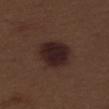{"biopsy_status": "not biopsied; imaged during a skin examination", "site": "right thigh", "patient": {"sex": "male", "age_approx": 70}, "automated_metrics": {"nevus_likeness_0_100": 90}, "image": {"source": "total-body photography crop", "field_of_view_mm": 15}, "lesion_size": {"long_diameter_mm_approx": 4.5}}